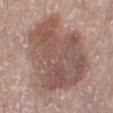biopsy status: catalogued during a skin exam; not biopsied | acquisition: ~15 mm crop, total-body skin-cancer survey | image-analysis metrics: a lesion area of about 65 mm², an eccentricity of roughly 0.45, and a shape-asymmetry score of about 0.3 (0 = symmetric); a lesion color around L≈53 a*≈18 b*≈23 in CIELAB, about 11 CIELAB-L* units darker than the surrounding skin, and a normalized lesion–skin contrast near 7.5; a border-irregularity index near 3.5/10 and a within-lesion color-variation index near 4.5/10; a nevus-likeness score of about 0/100 | site: the left lower leg | patient: female, roughly 65 years of age | size: about 10 mm.